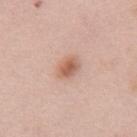follow-up: total-body-photography surveillance lesion; no biopsy
automated lesion analysis: a shape eccentricity near 0.8 and a shape-asymmetry score of about 0.2 (0 = symmetric); a lesion color around L≈60 a*≈22 b*≈30 in CIELAB and a lesion–skin lightness drop of about 11; a peripheral color-asymmetry measure near 1.5; a classifier nevus-likeness of about 90/100 and a lesion-detection confidence of about 100/100
size: ~3 mm (longest diameter)
site: the upper back
subject: female, about 65 years old
imaging modality: total-body-photography crop, ~15 mm field of view
illumination: white-light